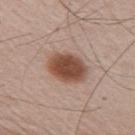This lesion was catalogued during total-body skin photography and was not selected for biopsy. A 15 mm close-up tile from a total-body photography series done for melanoma screening. The lesion is on the arm. Longest diameter approximately 4.5 mm. The subject is a male in their mid-60s. The total-body-photography lesion software estimated an average lesion color of about L≈49 a*≈20 b*≈27 (CIELAB), a lesion–skin lightness drop of about 15, and a lesion-to-skin contrast of about 11 (normalized; higher = more distinct). And it measured a border-irregularity index near 1/10, a color-variation rating of about 4.5/10, and peripheral color asymmetry of about 1. The tile uses white-light illumination.